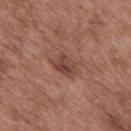The lesion was photographed on a routine skin check and not biopsied; there is no pathology result.
The subject is a male about 50 years old.
Approximately 3 mm at its widest.
On the mid back.
A close-up tile cropped from a whole-body skin photograph, about 15 mm across.
This is a white-light tile.